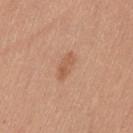Approximately 3.5 mm at its widest. An algorithmic analysis of the crop reported a lesion area of about 4 mm². The software also gave an average lesion color of about L≈57 a*≈22 b*≈33 (CIELAB) and a lesion-to-skin contrast of about 5.5 (normalized; higher = more distinct). The software also gave peripheral color asymmetry of about 1. The analysis additionally found a nevus-likeness score of about 45/100 and lesion-presence confidence of about 100/100. A female subject aged 38–42. Located on the left thigh. A 15 mm close-up tile from a total-body photography series done for melanoma screening. Captured under white-light illumination.